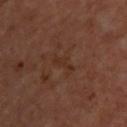Imaged during a routine full-body skin examination; the lesion was not biopsied and no histopathology is available.